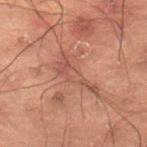Findings:
* workup · imaged on a skin check; not biopsied
* imaging modality · total-body-photography crop, ~15 mm field of view
* diameter · ≈5.5 mm
* image-analysis metrics · a lesion-to-skin contrast of about 5 (normalized; higher = more distinct); an automated nevus-likeness rating near 0 out of 100 and lesion-presence confidence of about 55/100
* patient · male, in their 50s
* anatomic site · the lower back